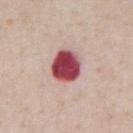Captured during whole-body skin photography for melanoma surveillance; the lesion was not biopsied. A roughly 15 mm field-of-view crop from a total-body skin photograph. A male subject approximately 60 years of age. About 4 mm across. Captured under white-light illumination. Automated image analysis of the tile measured a classifier nevus-likeness of about 0/100 and a detector confidence of about 100 out of 100 that the crop contains a lesion. Located on the chest.